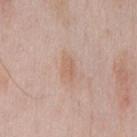{
  "biopsy_status": "not biopsied; imaged during a skin examination",
  "patient": {
    "sex": "male",
    "age_approx": 55
  },
  "image": {
    "source": "total-body photography crop",
    "field_of_view_mm": 15
  },
  "site": "chest"
}